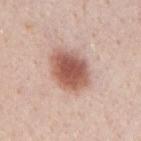From the mid back. A 15 mm close-up tile from a total-body photography series done for melanoma screening. The total-body-photography lesion software estimated a footprint of about 16 mm², a shape eccentricity near 0.7, and a symmetry-axis asymmetry near 0.15. It also reported a mean CIELAB color near L≈57 a*≈23 b*≈27, about 16 CIELAB-L* units darker than the surrounding skin, and a lesion-to-skin contrast of about 10 (normalized; higher = more distinct). And it measured a border-irregularity index near 1.5/10 and radial color variation of about 1.5. It also reported a nevus-likeness score of about 100/100. A male patient in their 40s.